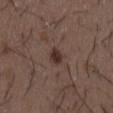Part of a total-body skin-imaging series; this lesion was reviewed on a skin check and was not flagged for biopsy.
From the back.
The subject is a male roughly 50 years of age.
This image is a 15 mm lesion crop taken from a total-body photograph.
The recorded lesion diameter is about 2.5 mm.
Imaged with white-light lighting.